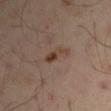{"biopsy_status": "not biopsied; imaged during a skin examination", "image": {"source": "total-body photography crop", "field_of_view_mm": 15}, "lighting": "cross-polarized", "site": "left upper arm", "lesion_size": {"long_diameter_mm_approx": 3.0}, "patient": {"sex": "male", "age_approx": 50}}